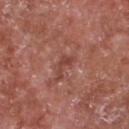The lesion was tiled from a total-body skin photograph and was not biopsied. Cropped from a whole-body photographic skin survey; the tile spans about 15 mm. The subject is a male aged 63–67. On the chest.